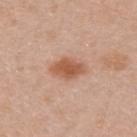The lesion was tiled from a total-body skin photograph and was not biopsied.
From the upper back.
A lesion tile, about 15 mm wide, cut from a 3D total-body photograph.
A male patient in their mid- to late 40s.